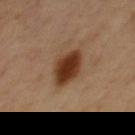Clinical impression: Part of a total-body skin-imaging series; this lesion was reviewed on a skin check and was not flagged for biopsy. Acquisition and patient details: The lesion's longest dimension is about 4.5 mm. A male subject, aged around 50. Automated tile analysis of the lesion measured an average lesion color of about L≈36 a*≈20 b*≈31 (CIELAB), a lesion–skin lightness drop of about 15, and a normalized border contrast of about 12.5. It also reported lesion-presence confidence of about 100/100. The lesion is located on the chest. A 15 mm close-up extracted from a 3D total-body photography capture.